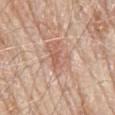Clinical impression: This lesion was catalogued during total-body skin photography and was not selected for biopsy. Acquisition and patient details: A male subject, aged around 80. The lesion is located on the mid back. A 15 mm crop from a total-body photograph taken for skin-cancer surveillance.